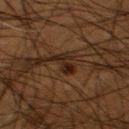This lesion was catalogued during total-body skin photography and was not selected for biopsy. The recorded lesion diameter is about 3 mm. Captured under cross-polarized illumination. The lesion is on the left thigh. Automated tile analysis of the lesion measured a lesion area of about 3.5 mm², an eccentricity of roughly 0.85, and two-axis asymmetry of about 0.5. The analysis additionally found a mean CIELAB color near L≈16 a*≈13 b*≈19. The software also gave border irregularity of about 6.5 on a 0–10 scale and a within-lesion color-variation index near 0.5/10. And it measured a lesion-detection confidence of about 95/100. Cropped from a total-body skin-imaging series; the visible field is about 15 mm. A male patient approximately 50 years of age.